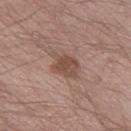The lesion was tiled from a total-body skin photograph and was not biopsied.
A male subject approximately 35 years of age.
The tile uses white-light illumination.
On the left thigh.
A 15 mm close-up tile from a total-body photography series done for melanoma screening.